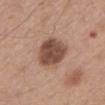Case summary:
• follow-up · catalogued during a skin exam; not biopsied
• lesion size · about 5 mm
• body site · the mid back
• image source · ~15 mm tile from a whole-body skin photo
• patient · male, roughly 55 years of age